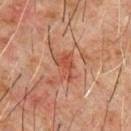<lesion>
  <biopsy_status>not biopsied; imaged during a skin examination</biopsy_status>
  <image>
    <source>total-body photography crop</source>
    <field_of_view_mm>15</field_of_view_mm>
  </image>
  <site>chest</site>
  <patient>
    <sex>male</sex>
    <age_approx>60</age_approx>
  </patient>
  <lesion_size>
    <long_diameter_mm_approx>3.5</long_diameter_mm_approx>
  </lesion_size>
</lesion>